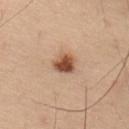Recorded during total-body skin imaging; not selected for excision or biopsy.
The tile uses cross-polarized illumination.
From the back.
An algorithmic analysis of the crop reported about 16 CIELAB-L* units darker than the surrounding skin and a normalized lesion–skin contrast near 10.5. And it measured internal color variation of about 4.5 on a 0–10 scale. And it measured a classifier nevus-likeness of about 100/100 and lesion-presence confidence of about 100/100.
Measured at roughly 2.5 mm in maximum diameter.
A 15 mm close-up tile from a total-body photography series done for melanoma screening.
A male subject, aged 53–57.